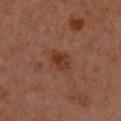Recorded during total-body skin imaging; not selected for excision or biopsy. Longest diameter approximately 3.5 mm. The patient is a female about 50 years old. From the right forearm. Automated image analysis of the tile measured a lesion color around L≈35 a*≈23 b*≈29 in CIELAB and a normalized border contrast of about 7.5. And it measured a border-irregularity rating of about 2.5/10, a color-variation rating of about 3/10, and peripheral color asymmetry of about 1. The software also gave a nevus-likeness score of about 40/100. A region of skin cropped from a whole-body photographic capture, roughly 15 mm wide. The tile uses cross-polarized illumination.